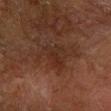<record>
  <biopsy_status>not biopsied; imaged during a skin examination</biopsy_status>
  <lighting>cross-polarized</lighting>
  <site>right forearm</site>
  <automated_metrics>
    <eccentricity>0.65</eccentricity>
    <shape_asymmetry>0.4</shape_asymmetry>
    <cielab_L>21</cielab_L>
    <cielab_a>16</cielab_a>
    <cielab_b>21</cielab_b>
    <vs_skin_darker_L>4.0</vs_skin_darker_L>
    <vs_skin_contrast_norm>5.5</vs_skin_contrast_norm>
  </automated_metrics>
  <patient>
    <sex>male</sex>
    <age_approx>60</age_approx>
  </patient>
  <image>
    <source>total-body photography crop</source>
    <field_of_view_mm>15</field_of_view_mm>
  </image>
  <lesion_size>
    <long_diameter_mm_approx>3.5</long_diameter_mm_approx>
  </lesion_size>
</record>